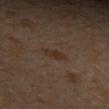workup = total-body-photography surveillance lesion; no biopsy | body site = the left upper arm | patient = male, in their mid-40s | tile lighting = cross-polarized | image source = ~15 mm tile from a whole-body skin photo | size = ~2.5 mm (longest diameter).